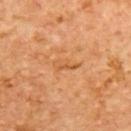A 15 mm crop from a total-body photograph taken for skin-cancer surveillance. On the upper back. The patient is a female aged around 55. About 3 mm across. Captured under cross-polarized illumination.